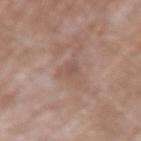– biopsy status — catalogued during a skin exam; not biopsied
– illumination — white-light illumination
– subject — male, aged around 60
– acquisition — ~15 mm crop, total-body skin-cancer survey
– site — the arm
– diameter — ~3 mm (longest diameter)